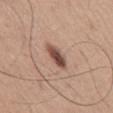notes — no biopsy performed (imaged during a skin exam); image — ~15 mm crop, total-body skin-cancer survey; site — the front of the torso; patient — male, about 65 years old.